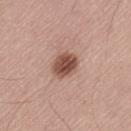follow-up — total-body-photography surveillance lesion; no biopsy
size — ≈3 mm
site — the leg
lighting — white-light
patient — male, aged 53–57
acquisition — total-body-photography crop, ~15 mm field of view